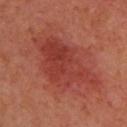workup: imaged on a skin check; not biopsied | subject: female, aged approximately 40 | body site: the upper back | automated metrics: a footprint of about 27 mm², an outline eccentricity of about 0.85 (0 = round, 1 = elongated), and a symmetry-axis asymmetry near 0.4; border irregularity of about 5.5 on a 0–10 scale, a within-lesion color-variation index near 4/10, and a peripheral color-asymmetry measure near 1.5 | illumination: cross-polarized illumination | image: ~15 mm tile from a whole-body skin photo.